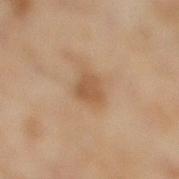workup = no biopsy performed (imaged during a skin exam)
image = 15 mm crop, total-body photography
lesion diameter = ≈3 mm
site = the right lower leg
patient = female, approximately 60 years of age